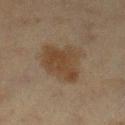• notes · catalogued during a skin exam; not biopsied
• patient · female, roughly 40 years of age
• image · total-body-photography crop, ~15 mm field of view
• site · the left lower leg